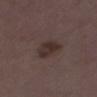<lesion>
<biopsy_status>not biopsied; imaged during a skin examination</biopsy_status>
<image>
  <source>total-body photography crop</source>
  <field_of_view_mm>15</field_of_view_mm>
</image>
<patient>
  <sex>female</sex>
  <age_approx>35</age_approx>
</patient>
<lesion_size>
  <long_diameter_mm_approx>4.0</long_diameter_mm_approx>
</lesion_size>
<site>left thigh</site>
</lesion>